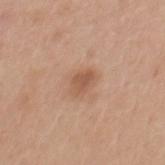follow-up: total-body-photography surveillance lesion; no biopsy
patient: male, aged 28–32
image-analysis metrics: a classifier nevus-likeness of about 40/100
lighting: white-light illumination
acquisition: 15 mm crop, total-body photography
location: the mid back
lesion diameter: about 3 mm